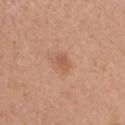This lesion was catalogued during total-body skin photography and was not selected for biopsy. This is a white-light tile. A female patient, roughly 40 years of age. About 2.5 mm across. A 15 mm crop from a total-body photograph taken for skin-cancer surveillance. From the chest.